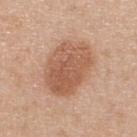Impression:
The lesion was tiled from a total-body skin photograph and was not biopsied.
Clinical summary:
A female subject, approximately 40 years of age. The total-body-photography lesion software estimated an average lesion color of about L≈57 a*≈22 b*≈32 (CIELAB), a lesion–skin lightness drop of about 11, and a normalized lesion–skin contrast near 7.5. Measured at roughly 6.5 mm in maximum diameter. The lesion is on the upper back. This is a white-light tile. A lesion tile, about 15 mm wide, cut from a 3D total-body photograph.